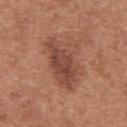biopsy status: imaged on a skin check; not biopsied | imaging modality: 15 mm crop, total-body photography | lesion diameter: about 7 mm | site: the abdomen | patient: male, about 65 years old.